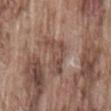follow-up: catalogued during a skin exam; not biopsied
subject: male, aged 73 to 77
acquisition: ~15 mm tile from a whole-body skin photo
diameter: about 5 mm
illumination: white-light illumination
automated metrics: a lesion area of about 10 mm², an outline eccentricity of about 0.8 (0 = round, 1 = elongated), and two-axis asymmetry of about 0.55; a lesion color around L≈48 a*≈18 b*≈25 in CIELAB, about 8 CIELAB-L* units darker than the surrounding skin, and a normalized lesion–skin contrast near 6; internal color variation of about 4 on a 0–10 scale and a peripheral color-asymmetry measure near 1
location: the lower back